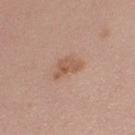body site: the leg | imaging modality: 15 mm crop, total-body photography | subject: female, aged around 30 | lesion diameter: ≈3.5 mm.